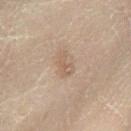Impression: Imaged during a routine full-body skin examination; the lesion was not biopsied and no histopathology is available. Context: Imaged with cross-polarized lighting. A region of skin cropped from a whole-body photographic capture, roughly 15 mm wide. A male subject aged 68 to 72. Located on the leg.